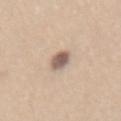The lesion was tiled from a total-body skin photograph and was not biopsied.